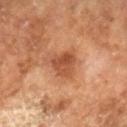{"biopsy_status": "not biopsied; imaged during a skin examination", "patient": {"sex": "male", "age_approx": 65}, "lighting": "cross-polarized", "image": {"source": "total-body photography crop", "field_of_view_mm": 15}}